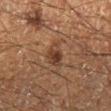The lesion was tiled from a total-body skin photograph and was not biopsied. A 15 mm crop from a total-body photograph taken for skin-cancer surveillance. The total-body-photography lesion software estimated an area of roughly 5 mm². It also reported an average lesion color of about L≈35 a*≈19 b*≈27 (CIELAB), about 9 CIELAB-L* units darker than the surrounding skin, and a normalized border contrast of about 8. The analysis additionally found a lesion-detection confidence of about 100/100. From the right lower leg. A male subject, in their 60s. Imaged with cross-polarized lighting.